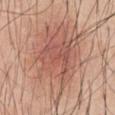Background:
The subject is a male in their mid-40s. Captured under white-light illumination. The recorded lesion diameter is about 10 mm. A roughly 15 mm field-of-view crop from a total-body skin photograph. Located on the chest.